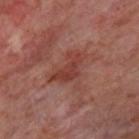The lesion was tiled from a total-body skin photograph and was not biopsied. The lesion is on the upper back. Cropped from a whole-body photographic skin survey; the tile spans about 15 mm. Imaged with cross-polarized lighting. The patient is roughly 55 years of age. Approximately 5 mm at its widest.